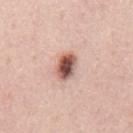Case summary:
- subject — male, approximately 55 years of age
- lesion diameter — about 3.5 mm
- TBP lesion metrics — a lesion area of about 6.5 mm², an outline eccentricity of about 0.75 (0 = round, 1 = elongated), and a shape-asymmetry score of about 0.2 (0 = symmetric); a border-irregularity index near 2/10, internal color variation of about 8 on a 0–10 scale, and a peripheral color-asymmetry measure near 2.5
- image source — ~15 mm crop, total-body skin-cancer survey
- anatomic site — the mid back
- lighting — white-light illumination